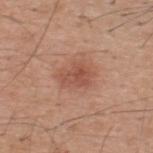Part of a total-body skin-imaging series; this lesion was reviewed on a skin check and was not flagged for biopsy. A male patient, aged around 60. A close-up tile cropped from a whole-body skin photograph, about 15 mm across. The lesion is on the upper back. An algorithmic analysis of the crop reported an area of roughly 7.5 mm² and an eccentricity of roughly 0.7. It also reported a mean CIELAB color near L≈52 a*≈24 b*≈29 and a lesion–skin lightness drop of about 9. The software also gave a border-irregularity index near 3.5/10. The software also gave a classifier nevus-likeness of about 75/100 and lesion-presence confidence of about 100/100.